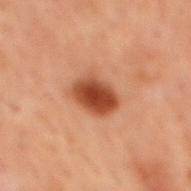No biopsy was performed on this lesion — it was imaged during a full skin examination and was not determined to be concerning. From the mid back. A male subject about 60 years old. A lesion tile, about 15 mm wide, cut from a 3D total-body photograph. The recorded lesion diameter is about 4 mm. The lesion-visualizer software estimated a mean CIELAB color near L≈36 a*≈23 b*≈29, a lesion–skin lightness drop of about 13, and a normalized lesion–skin contrast near 11. The software also gave a border-irregularity rating of about 2/10 and a peripheral color-asymmetry measure near 1. The analysis additionally found a classifier nevus-likeness of about 100/100 and lesion-presence confidence of about 100/100. Imaged with cross-polarized lighting.